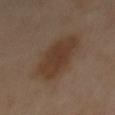workup: imaged on a skin check; not biopsied
image source: total-body-photography crop, ~15 mm field of view
image-analysis metrics: an area of roughly 20 mm² and an outline eccentricity of about 0.9 (0 = round, 1 = elongated); a border-irregularity index near 2.5/10, internal color variation of about 2.5 on a 0–10 scale, and peripheral color asymmetry of about 1
diameter: about 7.5 mm
body site: the mid back